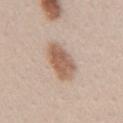Longest diameter approximately 5 mm. The total-body-photography lesion software estimated two-axis asymmetry of about 0.2. It also reported a normalized lesion–skin contrast near 8.5. Cropped from a total-body skin-imaging series; the visible field is about 15 mm. From the right upper arm. Imaged with white-light lighting. The patient is a female approximately 40 years of age.